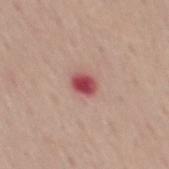Impression: The lesion was photographed on a routine skin check and not biopsied; there is no pathology result. Clinical summary: The patient is a male in their mid- to late 50s. The lesion is located on the mid back. About 2.5 mm across. Captured under white-light illumination. A region of skin cropped from a whole-body photographic capture, roughly 15 mm wide.